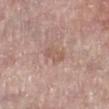This lesion was catalogued during total-body skin photography and was not selected for biopsy. Captured under white-light illumination. The patient is a female about 60 years old. The lesion is located on the right lower leg. Automated image analysis of the tile measured a lesion color around L≈56 a*≈19 b*≈26 in CIELAB, a lesion–skin lightness drop of about 7, and a normalized border contrast of about 5.5. The analysis additionally found a within-lesion color-variation index near 1/10 and a peripheral color-asymmetry measure near 0. Longest diameter approximately 3 mm. A 15 mm crop from a total-body photograph taken for skin-cancer surveillance.